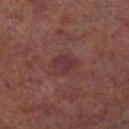notes: imaged on a skin check; not biopsied
anatomic site: the left lower leg
size: ~3 mm (longest diameter)
subject: male, approximately 65 years of age
lighting: cross-polarized
imaging modality: 15 mm crop, total-body photography